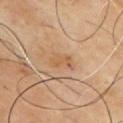Recorded during total-body skin imaging; not selected for excision or biopsy. The lesion is located on the chest. A 15 mm close-up tile from a total-body photography series done for melanoma screening. A male subject in their mid-60s.